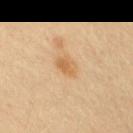Assessment:
The lesion was tiled from a total-body skin photograph and was not biopsied.
Image and clinical context:
A male subject, approximately 40 years of age. This is a cross-polarized tile. About 3 mm across. A region of skin cropped from a whole-body photographic capture, roughly 15 mm wide. Automated tile analysis of the lesion measured a normalized lesion–skin contrast near 6.5. And it measured a border-irregularity index near 2.5/10, a color-variation rating of about 2/10, and peripheral color asymmetry of about 0.5. On the right upper arm.